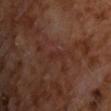follow-up: imaged on a skin check; not biopsied | subject: male, roughly 60 years of age | site: the back | tile lighting: cross-polarized illumination | acquisition: ~15 mm tile from a whole-body skin photo.